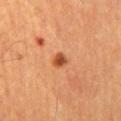Q: Was this lesion biopsied?
A: imaged on a skin check; not biopsied
Q: What is the anatomic site?
A: the abdomen
Q: How was the tile lit?
A: cross-polarized illumination
Q: What kind of image is this?
A: ~15 mm tile from a whole-body skin photo
Q: Patient demographics?
A: male, in their mid- to late 60s
Q: Automated lesion metrics?
A: border irregularity of about 1.5 on a 0–10 scale, a color-variation rating of about 5/10, and radial color variation of about 1.5
Q: Lesion size?
A: ~2 mm (longest diameter)